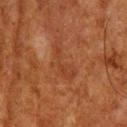Assessment:
No biopsy was performed on this lesion — it was imaged during a full skin examination and was not determined to be concerning.
Image and clinical context:
Measured at roughly 5.5 mm in maximum diameter. The patient is a male aged around 65. The lesion-visualizer software estimated a border-irregularity index near 9/10, internal color variation of about 1.5 on a 0–10 scale, and a peripheral color-asymmetry measure near 0.5. And it measured a nevus-likeness score of about 0/100 and a detector confidence of about 60 out of 100 that the crop contains a lesion. The lesion is on the head or neck. This image is a 15 mm lesion crop taken from a total-body photograph.